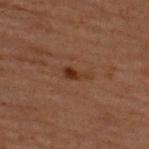<record>
  <patient>
    <sex>male</sex>
    <age_approx>60</age_approx>
  </patient>
  <site>upper back</site>
  <automated_metrics>
    <area_mm2_approx>3.5</area_mm2_approx>
    <eccentricity>0.9</eccentricity>
    <shape_asymmetry>0.35</shape_asymmetry>
    <cielab_L>25</cielab_L>
    <cielab_a>17</cielab_a>
    <cielab_b>24</cielab_b>
    <vs_skin_darker_L>6.0</vs_skin_darker_L>
    <nevus_likeness_0_100>30</nevus_likeness_0_100>
    <lesion_detection_confidence_0_100>100</lesion_detection_confidence_0_100>
  </automated_metrics>
  <image>
    <source>total-body photography crop</source>
    <field_of_view_mm>15</field_of_view_mm>
  </image>
  <lighting>cross-polarized</lighting>
  <lesion_size>
    <long_diameter_mm_approx>3.0</long_diameter_mm_approx>
  </lesion_size>
</record>